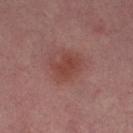Q: Is there a histopathology result?
A: imaged on a skin check; not biopsied
Q: How large is the lesion?
A: ~3.5 mm (longest diameter)
Q: What are the patient's age and sex?
A: female, aged 38 to 42
Q: What lighting was used for the tile?
A: cross-polarized
Q: What is the anatomic site?
A: the left thigh
Q: What is the imaging modality?
A: 15 mm crop, total-body photography